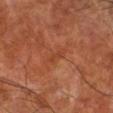{
  "biopsy_status": "not biopsied; imaged during a skin examination",
  "site": "right lower leg",
  "image": {
    "source": "total-body photography crop",
    "field_of_view_mm": 15
  },
  "patient": {
    "sex": "male",
    "age_approx": 60
  }
}